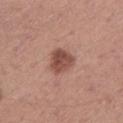diameter — about 3 mm
TBP lesion metrics — a lesion area of about 7.5 mm² and two-axis asymmetry of about 0.25; a border-irregularity rating of about 2.5/10, a color-variation rating of about 3.5/10, and peripheral color asymmetry of about 1.5
anatomic site — the left lower leg
image — 15 mm crop, total-body photography
subject — male, approximately 75 years of age
lighting — white-light